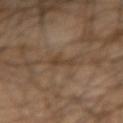Q: Was a biopsy performed?
A: imaged on a skin check; not biopsied
Q: Automated lesion metrics?
A: a lesion color around L≈40 a*≈14 b*≈28 in CIELAB, roughly 6 lightness units darker than nearby skin, and a normalized lesion–skin contrast near 5.5
Q: Lesion size?
A: ~3 mm (longest diameter)
Q: Patient demographics?
A: male, aged 63 to 67
Q: Lesion location?
A: the right forearm
Q: What is the imaging modality?
A: ~15 mm crop, total-body skin-cancer survey
Q: How was the tile lit?
A: cross-polarized illumination